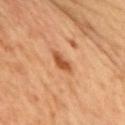Impression: The lesion was tiled from a total-body skin photograph and was not biopsied. Background: A 15 mm close-up tile from a total-body photography series done for melanoma screening. The subject is a male in their mid- to late 50s. This is a cross-polarized tile. The lesion is on the chest. An algorithmic analysis of the crop reported a footprint of about 4 mm² and a shape eccentricity near 0.8. The software also gave about 10 CIELAB-L* units darker than the surrounding skin and a lesion-to-skin contrast of about 8.5 (normalized; higher = more distinct).